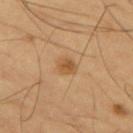| field | value |
|---|---|
| workup | no biopsy performed (imaged during a skin exam) |
| anatomic site | the left thigh |
| lighting | cross-polarized |
| TBP lesion metrics | a lesion area of about 4 mm²; an average lesion color of about L≈55 a*≈20 b*≈40 (CIELAB), a lesion–skin lightness drop of about 9, and a normalized lesion–skin contrast near 7; a border-irregularity index near 1.5/10 and radial color variation of about 1 |
| image | ~15 mm crop, total-body skin-cancer survey |
| subject | male, aged 63 to 67 |
| diameter | about 2.5 mm |